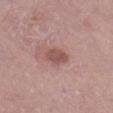Case summary:
- follow-up — no biopsy performed (imaged during a skin exam)
- image — 15 mm crop, total-body photography
- anatomic site — the leg
- subject — male, about 75 years old
- tile lighting — white-light illumination
- lesion size — ~3 mm (longest diameter)